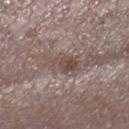The subject is a female aged around 40. A roughly 15 mm field-of-view crop from a total-body skin photograph. Measured at roughly 4 mm in maximum diameter. From the leg. The lesion-visualizer software estimated an average lesion color of about L≈47 a*≈15 b*≈20 (CIELAB), roughly 9 lightness units darker than nearby skin, and a normalized border contrast of about 7.